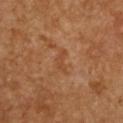Assessment:
Recorded during total-body skin imaging; not selected for excision or biopsy.
Acquisition and patient details:
A female patient, about 55 years old. A region of skin cropped from a whole-body photographic capture, roughly 15 mm wide. The lesion is located on the chest. Imaged with cross-polarized lighting. About 3 mm across.